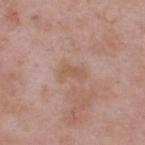Q: Is there a histopathology result?
A: imaged on a skin check; not biopsied
Q: How was this image acquired?
A: ~15 mm crop, total-body skin-cancer survey
Q: How large is the lesion?
A: ≈3 mm
Q: What is the anatomic site?
A: the upper back
Q: Illumination type?
A: white-light illumination
Q: Who is the patient?
A: male, in their mid-50s